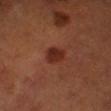illumination: cross-polarized | patient: male, aged 48 to 52 | image source: ~15 mm tile from a whole-body skin photo | image-analysis metrics: a lesion area of about 5 mm², an eccentricity of roughly 0.55, and two-axis asymmetry of about 0.2 | lesion diameter: about 2.5 mm | site: the right lower leg.